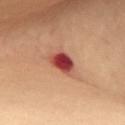biopsy_status: not biopsied; imaged during a skin examination
site: chest
patient:
  sex: female
  age_approx: 60
lighting: cross-polarized
image:
  source: total-body photography crop
  field_of_view_mm: 15
lesion_size:
  long_diameter_mm_approx: 3.5
automated_metrics:
  area_mm2_approx: 7.5
  eccentricity: 0.7
  shape_asymmetry: 0.3
  nevus_likeness_0_100: 0
  lesion_detection_confidence_0_100: 100